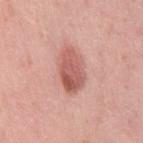image: ~15 mm crop, total-body skin-cancer survey
anatomic site: the right thigh
diameter: ≈5 mm
illumination: white-light illumination
patient: female, approximately 40 years of age
pathology: a dysplastic (Clark) nevus